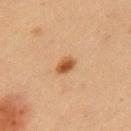{
  "biopsy_status": "not biopsied; imaged during a skin examination",
  "image": {
    "source": "total-body photography crop",
    "field_of_view_mm": 15
  },
  "patient": {
    "sex": "male",
    "age_approx": 60
  },
  "lesion_size": {
    "long_diameter_mm_approx": 2.5
  },
  "site": "left upper arm",
  "automated_metrics": {
    "cielab_L": 45,
    "cielab_a": 20,
    "cielab_b": 33,
    "vs_skin_darker_L": 11.0,
    "vs_skin_contrast_norm": 9.0,
    "nevus_likeness_0_100": 100,
    "lesion_detection_confidence_0_100": 100
  },
  "lighting": "cross-polarized"
}